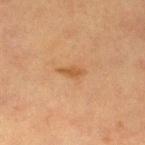{
  "biopsy_status": "not biopsied; imaged during a skin examination",
  "lighting": "cross-polarized",
  "automated_metrics": {
    "cielab_L": 45,
    "cielab_a": 19,
    "cielab_b": 34,
    "vs_skin_darker_L": 7.0,
    "vs_skin_contrast_norm": 6.5,
    "color_variation_0_10": 0.5,
    "peripheral_color_asymmetry": 0.0
  },
  "image": {
    "source": "total-body photography crop",
    "field_of_view_mm": 15
  },
  "lesion_size": {
    "long_diameter_mm_approx": 3.0
  },
  "patient": {
    "sex": "female",
    "age_approx": 55
  },
  "site": "left lower leg"
}